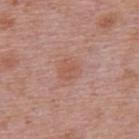Located on the upper back.
Imaged with white-light lighting.
A 15 mm close-up extracted from a 3D total-body photography capture.
Longest diameter approximately 2.5 mm.
Automated image analysis of the tile measured a mean CIELAB color near L≈55 a*≈23 b*≈28, about 6 CIELAB-L* units darker than the surrounding skin, and a normalized border contrast of about 5. The analysis additionally found a classifier nevus-likeness of about 5/100 and a lesion-detection confidence of about 100/100.
A male patient, aged 73–77.